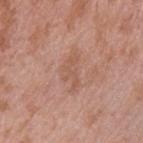Part of a total-body skin-imaging series; this lesion was reviewed on a skin check and was not flagged for biopsy.
The subject is a male aged 38 to 42.
A 15 mm close-up tile from a total-body photography series done for melanoma screening.
Imaged with white-light lighting.
From the arm.
Longest diameter approximately 4.5 mm.